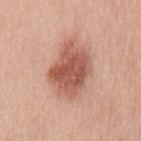biopsy status: no biopsy performed (imaged during a skin exam)
location: the mid back
diameter: about 6.5 mm
tile lighting: white-light
acquisition: total-body-photography crop, ~15 mm field of view
subject: female, about 40 years old
TBP lesion metrics: a mean CIELAB color near L≈57 a*≈24 b*≈29, a lesion–skin lightness drop of about 14, and a normalized border contrast of about 9; a border-irregularity index near 2.5/10, a color-variation rating of about 5/10, and a peripheral color-asymmetry measure near 1.5